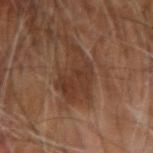Notes:
• biopsy status: no biopsy performed (imaged during a skin exam)
• body site: the right arm
• illumination: cross-polarized
• lesion diameter: about 5.5 mm
• image: total-body-photography crop, ~15 mm field of view
• patient: male, aged 58 to 62